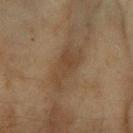Impression:
No biopsy was performed on this lesion — it was imaged during a full skin examination and was not determined to be concerning.
Background:
About 6 mm across. The lesion is located on the right forearm. Automated image analysis of the tile measured a lesion area of about 11 mm², an outline eccentricity of about 0.9 (0 = round, 1 = elongated), and a shape-asymmetry score of about 0.3 (0 = symmetric). The software also gave border irregularity of about 4 on a 0–10 scale and a peripheral color-asymmetry measure near 1. The subject is a female aged approximately 60. A close-up tile cropped from a whole-body skin photograph, about 15 mm across. Imaged with cross-polarized lighting.